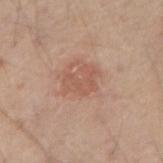Q: Is there a histopathology result?
A: catalogued during a skin exam; not biopsied
Q: How was the tile lit?
A: white-light illumination
Q: Patient demographics?
A: male, about 45 years old
Q: Lesion size?
A: ~3.5 mm (longest diameter)
Q: Lesion location?
A: the right forearm
Q: What is the imaging modality?
A: total-body-photography crop, ~15 mm field of view